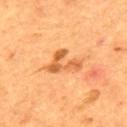Imaged during a routine full-body skin examination; the lesion was not biopsied and no histopathology is available. A male patient, approximately 55 years of age. Located on the upper back. Longest diameter approximately 4.5 mm. This is a cross-polarized tile. Automated tile analysis of the lesion measured a lesion color around L≈58 a*≈27 b*≈44 in CIELAB, a lesion–skin lightness drop of about 11, and a normalized lesion–skin contrast near 7.5. And it measured a classifier nevus-likeness of about 0/100 and a detector confidence of about 100 out of 100 that the crop contains a lesion. A 15 mm close-up tile from a total-body photography series done for melanoma screening.